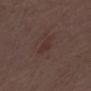Imaged during a routine full-body skin examination; the lesion was not biopsied and no histopathology is available.
On the left lower leg.
A male patient aged approximately 70.
The lesion's longest dimension is about 2.5 mm.
Cropped from a whole-body photographic skin survey; the tile spans about 15 mm.